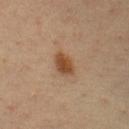Captured during whole-body skin photography for melanoma surveillance; the lesion was not biopsied. The lesion is located on the chest. This image is a 15 mm lesion crop taken from a total-body photograph. The subject is a male approximately 35 years of age.